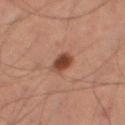lesion size — about 2.5 mm | illumination — cross-polarized | subject — male, approximately 60 years of age | site — the right thigh | imaging modality — ~15 mm tile from a whole-body skin photo.